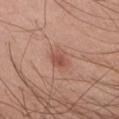This lesion was catalogued during total-body skin photography and was not selected for biopsy.
From the chest.
The lesion-visualizer software estimated an outline eccentricity of about 0.55 (0 = round, 1 = elongated) and a shape-asymmetry score of about 0.2 (0 = symmetric). And it measured a mean CIELAB color near L≈53 a*≈24 b*≈29, roughly 9 lightness units darker than nearby skin, and a lesion-to-skin contrast of about 6.5 (normalized; higher = more distinct). The software also gave a classifier nevus-likeness of about 80/100 and a detector confidence of about 100 out of 100 that the crop contains a lesion.
The tile uses white-light illumination.
A roughly 15 mm field-of-view crop from a total-body skin photograph.
A male subject, aged 38 to 42.